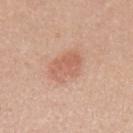Q: What is the lesion's diameter?
A: ~4 mm (longest diameter)
Q: What is the anatomic site?
A: the mid back
Q: How was the tile lit?
A: white-light illumination
Q: What is the imaging modality?
A: 15 mm crop, total-body photography
Q: What are the patient's age and sex?
A: male, about 45 years old